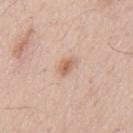TBP lesion metrics = an area of roughly 3.5 mm²; an average lesion color of about L≈63 a*≈19 b*≈31 (CIELAB), roughly 10 lightness units darker than nearby skin, and a normalized border contrast of about 7; internal color variation of about 3 on a 0–10 scale and a peripheral color-asymmetry measure near 1
lighting = white-light
body site = the mid back
patient = male, about 55 years old
image source = total-body-photography crop, ~15 mm field of view